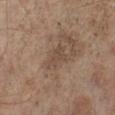Impression: Captured during whole-body skin photography for melanoma surveillance; the lesion was not biopsied.